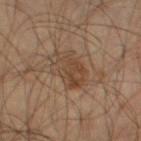No biopsy was performed on this lesion — it was imaged during a full skin examination and was not determined to be concerning.
A male patient in their mid-50s.
A region of skin cropped from a whole-body photographic capture, roughly 15 mm wide.
Imaged with cross-polarized lighting.
From the left thigh.
The total-body-photography lesion software estimated a border-irregularity rating of about 2.5/10 and internal color variation of about 3.5 on a 0–10 scale. And it measured a nevus-likeness score of about 30/100.